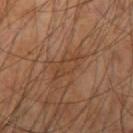workup: catalogued during a skin exam; not biopsied | patient: male, in their 60s | image-analysis metrics: a lesion area of about 5.5 mm² and a symmetry-axis asymmetry near 0.3; a nevus-likeness score of about 0/100 | lesion diameter: ~4 mm (longest diameter) | anatomic site: the right upper arm | image source: total-body-photography crop, ~15 mm field of view.